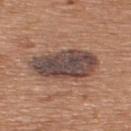No biopsy was performed on this lesion — it was imaged during a full skin examination and was not determined to be concerning.